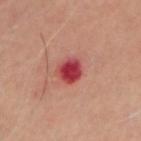Assessment:
No biopsy was performed on this lesion — it was imaged during a full skin examination and was not determined to be concerning.
Background:
The total-body-photography lesion software estimated a mean CIELAB color near L≈45 a*≈42 b*≈26, about 16 CIELAB-L* units darker than the surrounding skin, and a normalized lesion–skin contrast near 11.5. It also reported border irregularity of about 1.5 on a 0–10 scale, internal color variation of about 3.5 on a 0–10 scale, and a peripheral color-asymmetry measure near 1. And it measured an automated nevus-likeness rating near 0 out of 100 and a lesion-detection confidence of about 100/100. About 2.5 mm across. The subject is a male aged 53–57. Located on the head or neck. Cropped from a whole-body photographic skin survey; the tile spans about 15 mm.